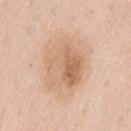Case summary:
• biopsy status · no biopsy performed (imaged during a skin exam)